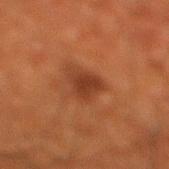follow-up: imaged on a skin check; not biopsied
size: ≈3 mm
patient: male, aged 63–67
imaging modality: ~15 mm tile from a whole-body skin photo
location: the right lower leg